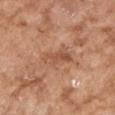Imaged during a routine full-body skin examination; the lesion was not biopsied and no histopathology is available.